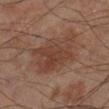Image and clinical context:
From the right lower leg. A region of skin cropped from a whole-body photographic capture, roughly 15 mm wide. Longest diameter approximately 6 mm. The subject is a male about 65 years old. Imaged with cross-polarized lighting.